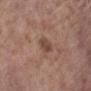Recorded during total-body skin imaging; not selected for excision or biopsy.
About 3 mm across.
This is a white-light tile.
The total-body-photography lesion software estimated an average lesion color of about L≈46 a*≈19 b*≈26 (CIELAB), a lesion–skin lightness drop of about 9, and a lesion-to-skin contrast of about 7 (normalized; higher = more distinct). The analysis additionally found a border-irregularity index near 2/10 and peripheral color asymmetry of about 0.5. The software also gave a classifier nevus-likeness of about 0/100 and a detector confidence of about 100 out of 100 that the crop contains a lesion.
The lesion is on the left lower leg.
A region of skin cropped from a whole-body photographic capture, roughly 15 mm wide.
A female subject aged 83 or older.